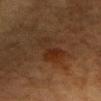<lesion>
<biopsy_status>not biopsied; imaged during a skin examination</biopsy_status>
<automated_metrics>
  <area_mm2_approx>9.0</area_mm2_approx>
  <eccentricity>0.9</eccentricity>
  <cielab_L>23</cielab_L>
  <cielab_a>16</cielab_a>
  <cielab_b>24</cielab_b>
  <vs_skin_darker_L>5.0</vs_skin_darker_L>
  <vs_skin_contrast_norm>6.0</vs_skin_contrast_norm>
  <peripheral_color_asymmetry>1.5</peripheral_color_asymmetry>
  <nevus_likeness_0_100>10</nevus_likeness_0_100>
  <lesion_detection_confidence_0_100>100</lesion_detection_confidence_0_100>
</automated_metrics>
<image>
  <source>total-body photography crop</source>
  <field_of_view_mm>15</field_of_view_mm>
</image>
<site>head or neck</site>
<patient>
  <sex>female</sex>
  <age_approx>55</age_approx>
</patient>
</lesion>